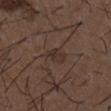No biopsy was performed on this lesion — it was imaged during a full skin examination and was not determined to be concerning.
The subject is a male about 50 years old.
The lesion is located on the chest.
A 15 mm close-up tile from a total-body photography series done for melanoma screening.
Imaged with white-light lighting.